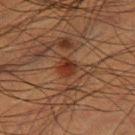Q: Was a biopsy performed?
A: catalogued during a skin exam; not biopsied
Q: What is the anatomic site?
A: the right thigh
Q: Who is the patient?
A: male, aged 48–52
Q: Lesion size?
A: about 2.5 mm
Q: Illumination type?
A: cross-polarized illumination
Q: What kind of image is this?
A: ~15 mm tile from a whole-body skin photo